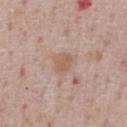Clinical impression: The lesion was tiled from a total-body skin photograph and was not biopsied. Clinical summary: The subject is a male aged around 75. Approximately 2.5 mm at its widest. The lesion is located on the chest. A roughly 15 mm field-of-view crop from a total-body skin photograph. Captured under white-light illumination. An algorithmic analysis of the crop reported a lesion–skin lightness drop of about 7 and a normalized lesion–skin contrast near 6.5. The software also gave a peripheral color-asymmetry measure near 0.5. It also reported an automated nevus-likeness rating near 25 out of 100.